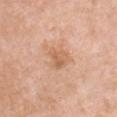follow-up: catalogued during a skin exam; not biopsied
image: ~15 mm tile from a whole-body skin photo
illumination: white-light illumination
subject: female, aged 68 to 72
diameter: about 3 mm
automated lesion analysis: a mean CIELAB color near L≈62 a*≈22 b*≈34, a lesion–skin lightness drop of about 8, and a lesion-to-skin contrast of about 5.5 (normalized; higher = more distinct)
body site: the chest